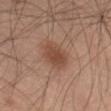biopsy status: no biopsy performed (imaged during a skin exam)
TBP lesion metrics: about 8 CIELAB-L* units darker than the surrounding skin and a lesion-to-skin contrast of about 7 (normalized; higher = more distinct); a border-irregularity index near 1.5/10, internal color variation of about 2.5 on a 0–10 scale, and peripheral color asymmetry of about 1
lighting: cross-polarized
lesion size: about 4 mm
acquisition: 15 mm crop, total-body photography
patient: male, in their mid- to late 50s
anatomic site: the leg